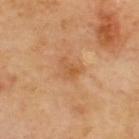Clinical impression: Imaged during a routine full-body skin examination; the lesion was not biopsied and no histopathology is available. Clinical summary: On the upper back. A lesion tile, about 15 mm wide, cut from a 3D total-body photograph. About 3 mm across.